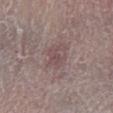The lesion was photographed on a routine skin check and not biopsied; there is no pathology result.
The tile uses white-light illumination.
A male patient, roughly 65 years of age.
A 15 mm close-up extracted from a 3D total-body photography capture.
About 3.5 mm across.
Located on the left lower leg.